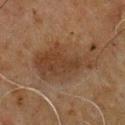Captured during whole-body skin photography for melanoma surveillance; the lesion was not biopsied. A male subject, in their 60s. About 8 mm across. A 15 mm crop from a total-body photograph taken for skin-cancer surveillance. Imaged with cross-polarized lighting. The lesion is located on the chest. Automated image analysis of the tile measured a border-irregularity rating of about 5.5/10, a color-variation rating of about 3.5/10, and radial color variation of about 1.5. And it measured a classifier nevus-likeness of about 10/100 and a detector confidence of about 95 out of 100 that the crop contains a lesion.